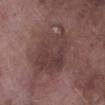workup = total-body-photography surveillance lesion; no biopsy | tile lighting = white-light illumination | imaging modality = 15 mm crop, total-body photography | subject = male, aged approximately 75 | lesion size = ≈7.5 mm | automated metrics = a lesion color around L≈39 a*≈18 b*≈18 in CIELAB, roughly 7 lightness units darker than nearby skin, and a normalized lesion–skin contrast near 6.5; internal color variation of about 4 on a 0–10 scale and peripheral color asymmetry of about 1.5 | body site = the right lower leg.